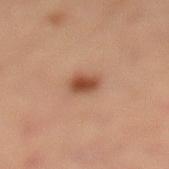Impression: This lesion was catalogued during total-body skin photography and was not selected for biopsy. Background: The patient is a female aged around 45. A 15 mm close-up extracted from a 3D total-body photography capture. Imaged with cross-polarized lighting. From the left lower leg. The total-body-photography lesion software estimated a lesion area of about 4.5 mm², an eccentricity of roughly 0.7, and a shape-asymmetry score of about 0.25 (0 = symmetric). It also reported a lesion–skin lightness drop of about 13 and a normalized lesion–skin contrast near 9.5. The analysis additionally found a color-variation rating of about 3/10 and a peripheral color-asymmetry measure near 1. It also reported a nevus-likeness score of about 100/100. The recorded lesion diameter is about 3 mm.